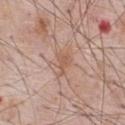biopsy status: no biopsy performed (imaged during a skin exam)
anatomic site: the front of the torso
tile lighting: white-light
size: about 4 mm
image source: ~15 mm tile from a whole-body skin photo
subject: male, in their 70s